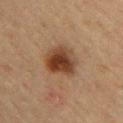location = the upper back | image = total-body-photography crop, ~15 mm field of view | automated metrics = a mean CIELAB color near L≈37 a*≈19 b*≈29, roughly 12 lightness units darker than nearby skin, and a normalized lesion–skin contrast near 10.5 | patient = male, approximately 40 years of age.